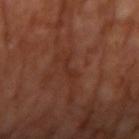Q: Was this lesion biopsied?
A: total-body-photography surveillance lesion; no biopsy
Q: Patient demographics?
A: male, aged 53 to 57
Q: Automated lesion metrics?
A: an area of roughly 2 mm², a shape eccentricity near 0.95, and a shape-asymmetry score of about 0.55 (0 = symmetric); an average lesion color of about L≈29 a*≈23 b*≈27 (CIELAB), a lesion–skin lightness drop of about 5, and a lesion-to-skin contrast of about 5 (normalized; higher = more distinct); a border-irregularity rating of about 6.5/10 and a peripheral color-asymmetry measure near 0
Q: Lesion size?
A: ~3 mm (longest diameter)
Q: Where on the body is the lesion?
A: the right upper arm
Q: What is the imaging modality?
A: 15 mm crop, total-body photography